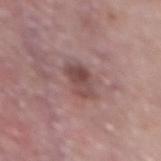{
  "biopsy_status": "not biopsied; imaged during a skin examination",
  "lesion_size": {
    "long_diameter_mm_approx": 3.5
  },
  "site": "mid back",
  "image": {
    "source": "total-body photography crop",
    "field_of_view_mm": 15
  },
  "patient": {
    "sex": "male",
    "age_approx": 70
  },
  "automated_metrics": {
    "area_mm2_approx": 6.5,
    "eccentricity": 0.8,
    "shape_asymmetry": 0.25,
    "cielab_L": 47,
    "cielab_a": 20,
    "cielab_b": 20,
    "vs_skin_darker_L": 10.0,
    "vs_skin_contrast_norm": 7.5,
    "border_irregularity_0_10": 2.5,
    "color_variation_0_10": 6.0,
    "peripheral_color_asymmetry": 2.5
  },
  "lighting": "white-light"
}